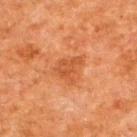Q: Was this lesion biopsied?
A: total-body-photography surveillance lesion; no biopsy
Q: Automated lesion metrics?
A: a border-irregularity index near 5/10 and internal color variation of about 2.5 on a 0–10 scale; a classifier nevus-likeness of about 0/100 and a lesion-detection confidence of about 100/100
Q: What is the imaging modality?
A: ~15 mm tile from a whole-body skin photo
Q: Lesion location?
A: the back
Q: What are the patient's age and sex?
A: male, aged 58–62